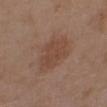Image and clinical context: A female subject, about 30 years old. From the left upper arm. Measured at roughly 4 mm in maximum diameter. A close-up tile cropped from a whole-body skin photograph, about 15 mm across. The tile uses white-light illumination.